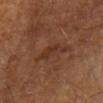Part of a total-body skin-imaging series; this lesion was reviewed on a skin check and was not flagged for biopsy. The total-body-photography lesion software estimated a mean CIELAB color near L≈32 a*≈22 b*≈28 and a lesion–skin lightness drop of about 6. A male subject, approximately 70 years of age. Longest diameter approximately 4 mm. From the arm. A region of skin cropped from a whole-body photographic capture, roughly 15 mm wide. Imaged with cross-polarized lighting.